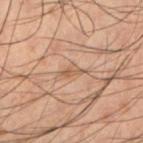– biopsy status: total-body-photography surveillance lesion; no biopsy
– illumination: cross-polarized illumination
– anatomic site: the left lower leg
– diameter: about 2.5 mm
– image-analysis metrics: border irregularity of about 4 on a 0–10 scale; an automated nevus-likeness rating near 0 out of 100 and a detector confidence of about 70 out of 100 that the crop contains a lesion
– subject: male, aged 48 to 52
– acquisition: 15 mm crop, total-body photography